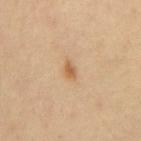This lesion was catalogued during total-body skin photography and was not selected for biopsy.
The lesion's longest dimension is about 2 mm.
Located on the back.
A lesion tile, about 15 mm wide, cut from a 3D total-body photograph.
Captured under cross-polarized illumination.
A male patient, aged 58–62.